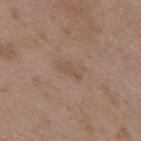biopsy_status: not biopsied; imaged during a skin examination
automated_metrics:
  vs_skin_darker_L: 5.0
  vs_skin_contrast_norm: 4.5
  border_irregularity_0_10: 5.0
  color_variation_0_10: 0.0
  peripheral_color_asymmetry: 0.0
lesion_size:
  long_diameter_mm_approx: 3.0
patient:
  sex: male
  age_approx: 50
site: mid back
image:
  source: total-body photography crop
  field_of_view_mm: 15
lighting: white-light